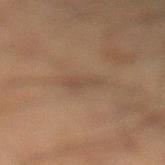follow-up — total-body-photography surveillance lesion; no biopsy | TBP lesion metrics — an area of roughly 4 mm² and an outline eccentricity of about 0.85 (0 = round, 1 = elongated); an average lesion color of about L≈40 a*≈14 b*≈24 (CIELAB), a lesion–skin lightness drop of about 5, and a lesion-to-skin contrast of about 4.5 (normalized; higher = more distinct); a border-irregularity rating of about 3/10, internal color variation of about 2 on a 0–10 scale, and peripheral color asymmetry of about 0.5; an automated nevus-likeness rating near 0 out of 100 and a detector confidence of about 100 out of 100 that the crop contains a lesion | illumination — cross-polarized | acquisition — ~15 mm crop, total-body skin-cancer survey | patient — male, aged 53 to 57 | body site — the right lower leg | diameter — ≈3 mm.